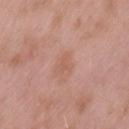No biopsy was performed on this lesion — it was imaged during a full skin examination and was not determined to be concerning.
On the back.
A close-up tile cropped from a whole-body skin photograph, about 15 mm across.
Approximately 2.5 mm at its widest.
The patient is a male roughly 55 years of age.